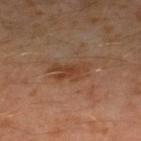Captured during whole-body skin photography for melanoma surveillance; the lesion was not biopsied.
Captured under cross-polarized illumination.
This image is a 15 mm lesion crop taken from a total-body photograph.
A male subject, in their 30s.
Measured at roughly 4.5 mm in maximum diameter.
Located on the left leg.
Automated image analysis of the tile measured an average lesion color of about L≈40 a*≈20 b*≈31 (CIELAB), roughly 8 lightness units darker than nearby skin, and a normalized lesion–skin contrast near 7.